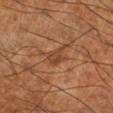biopsy_status: not biopsied; imaged during a skin examination
image:
  source: total-body photography crop
  field_of_view_mm: 15
site: right lower leg
patient:
  sex: male
  age_approx: 70